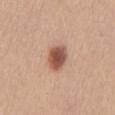Captured during whole-body skin photography for melanoma surveillance; the lesion was not biopsied. The lesion is located on the mid back. A female patient roughly 40 years of age. The total-body-photography lesion software estimated a classifier nevus-likeness of about 100/100. A close-up tile cropped from a whole-body skin photograph, about 15 mm across. This is a white-light tile. Measured at roughly 3.5 mm in maximum diameter.